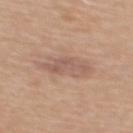subject=female, aged 53–57; image=15 mm crop, total-body photography; site=the back.